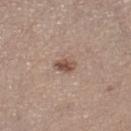– notes — no biopsy performed (imaged during a skin exam)
– image — 15 mm crop, total-body photography
– diameter — ~2.5 mm (longest diameter)
– lighting — white-light illumination
– patient — female, in their mid-60s
– body site — the right lower leg
– image-analysis metrics — a lesion color around L≈50 a*≈19 b*≈26 in CIELAB, a lesion–skin lightness drop of about 12, and a normalized border contrast of about 9; border irregularity of about 2.5 on a 0–10 scale, a color-variation rating of about 2.5/10, and a peripheral color-asymmetry measure near 1